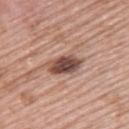Q: Was a biopsy performed?
A: catalogued during a skin exam; not biopsied
Q: What is the imaging modality?
A: ~15 mm tile from a whole-body skin photo
Q: What is the anatomic site?
A: the upper back
Q: Who is the patient?
A: female, aged 58 to 62